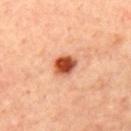The lesion was tiled from a total-body skin photograph and was not biopsied. A 15 mm close-up tile from a total-body photography series done for melanoma screening. A female patient, aged approximately 45. The tile uses cross-polarized illumination. Measured at roughly 2.5 mm in maximum diameter. The total-body-photography lesion software estimated a border-irregularity rating of about 1/10 and peripheral color asymmetry of about 1.5. The lesion is located on the upper back.